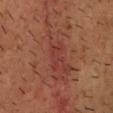Clinical impression:
Imaged during a routine full-body skin examination; the lesion was not biopsied and no histopathology is available.
Background:
Longest diameter approximately 4 mm. The lesion is on the head or neck. The tile uses cross-polarized illumination. A male subject aged around 50. A close-up tile cropped from a whole-body skin photograph, about 15 mm across.